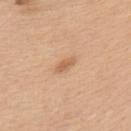Clinical impression:
No biopsy was performed on this lesion — it was imaged during a full skin examination and was not determined to be concerning.
Background:
Longest diameter approximately 2.5 mm. From the upper back. The tile uses white-light illumination. This image is a 15 mm lesion crop taken from a total-body photograph. The lesion-visualizer software estimated a border-irregularity index near 3.5/10 and peripheral color asymmetry of about 0. The analysis additionally found a classifier nevus-likeness of about 55/100 and a detector confidence of about 100 out of 100 that the crop contains a lesion. A male patient, aged approximately 60.